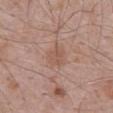Q: Is there a histopathology result?
A: catalogued during a skin exam; not biopsied
Q: What kind of image is this?
A: total-body-photography crop, ~15 mm field of view
Q: What did automated image analysis measure?
A: a lesion-detection confidence of about 100/100
Q: How large is the lesion?
A: ≈2.5 mm
Q: Who is the patient?
A: male, aged approximately 70
Q: What is the anatomic site?
A: the abdomen
Q: Illumination type?
A: white-light illumination